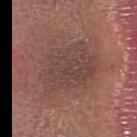notes = imaged on a skin check; not biopsied | anatomic site = the head or neck | acquisition = 15 mm crop, total-body photography | subject = female, roughly 40 years of age.